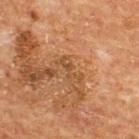Assessment: Captured during whole-body skin photography for melanoma surveillance; the lesion was not biopsied. Clinical summary: A roughly 15 mm field-of-view crop from a total-body skin photograph. The subject is a male aged approximately 65. The lesion's longest dimension is about 3.5 mm. From the back.